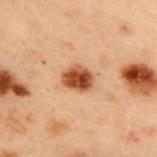- biopsy status: imaged on a skin check; not biopsied
- diameter: ≈3.5 mm
- lighting: cross-polarized
- image: ~15 mm crop, total-body skin-cancer survey
- subject: male, roughly 55 years of age
- location: the upper back